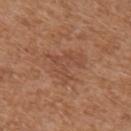Imaged during a routine full-body skin examination; the lesion was not biopsied and no histopathology is available. The lesion is located on the right upper arm. The total-body-photography lesion software estimated a border-irregularity index near 7/10, a within-lesion color-variation index near 2/10, and a peripheral color-asymmetry measure near 1. The analysis additionally found a classifier nevus-likeness of about 0/100 and a detector confidence of about 100 out of 100 that the crop contains a lesion. About 5 mm across. A female patient, aged approximately 40. The tile uses white-light illumination. Cropped from a total-body skin-imaging series; the visible field is about 15 mm.